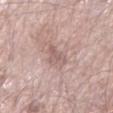  biopsy_status: not biopsied; imaged during a skin examination
  patient:
    sex: male
    age_approx: 60
  lesion_size:
    long_diameter_mm_approx: 3.5
  image:
    source: total-body photography crop
    field_of_view_mm: 15
  site: left forearm